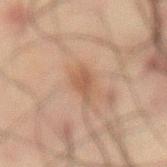Case summary:
– biopsy status — no biopsy performed (imaged during a skin exam)
– TBP lesion metrics — a within-lesion color-variation index near 1.5/10 and peripheral color asymmetry of about 0.5; lesion-presence confidence of about 100/100
– lesion size — about 3.5 mm
– image source — 15 mm crop, total-body photography
– tile lighting — cross-polarized illumination
– site — the abdomen
– subject — male, aged 58 to 62